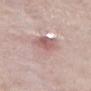Clinical impression: The lesion was photographed on a routine skin check and not biopsied; there is no pathology result. Clinical summary: The lesion's longest dimension is about 5 mm. Cropped from a whole-body photographic skin survey; the tile spans about 15 mm. A male subject about 55 years old. Located on the abdomen.